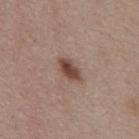Assessment: No biopsy was performed on this lesion — it was imaged during a full skin examination and was not determined to be concerning. Context: The lesion is on the mid back. Approximately 3.5 mm at its widest. Captured under white-light illumination. A 15 mm close-up extracted from a 3D total-body photography capture. A female subject approximately 45 years of age.